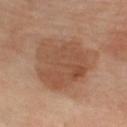{
  "biopsy_status": "not biopsied; imaged during a skin examination",
  "lighting": "cross-polarized",
  "patient": {
    "sex": "female",
    "age_approx": 50
  },
  "lesion_size": {
    "long_diameter_mm_approx": 7.5
  },
  "image": {
    "source": "total-body photography crop",
    "field_of_view_mm": 15
  },
  "site": "right lower leg"
}